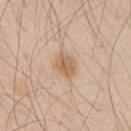Findings:
– notes · imaged on a skin check; not biopsied
– image source · 15 mm crop, total-body photography
– diameter · ≈3 mm
– patient · male, aged 43 to 47
– illumination · white-light
– body site · the right upper arm
– automated metrics · a mean CIELAB color near L≈61 a*≈17 b*≈33, roughly 10 lightness units darker than nearby skin, and a normalized lesion–skin contrast near 7.5